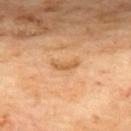Image and clinical context: A 15 mm crop from a total-body photograph taken for skin-cancer surveillance. From the back. Captured under cross-polarized illumination. The recorded lesion diameter is about 3 mm. A male subject, aged 68 to 72. An algorithmic analysis of the crop reported an area of roughly 3 mm², an outline eccentricity of about 0.95 (0 = round, 1 = elongated), and a shape-asymmetry score of about 0.55 (0 = symmetric).